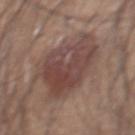No biopsy was performed on this lesion — it was imaged during a full skin examination and was not determined to be concerning.
The lesion's longest dimension is about 7 mm.
From the abdomen.
A region of skin cropped from a whole-body photographic capture, roughly 15 mm wide.
A male patient, in their 60s.